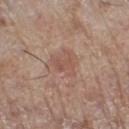Findings:
- notes · no biopsy performed (imaged during a skin exam)
- lesion diameter · ~3.5 mm (longest diameter)
- body site · the leg
- illumination · white-light
- image · total-body-photography crop, ~15 mm field of view
- automated lesion analysis · a shape eccentricity near 0.65 and two-axis asymmetry of about 0.4; a lesion color around L≈52 a*≈20 b*≈26 in CIELAB, about 7 CIELAB-L* units darker than the surrounding skin, and a normalized border contrast of about 5; border irregularity of about 5 on a 0–10 scale, a color-variation rating of about 3/10, and a peripheral color-asymmetry measure near 1; a nevus-likeness score of about 0/100
- subject · male, aged around 70